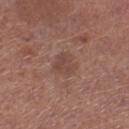This lesion was catalogued during total-body skin photography and was not selected for biopsy.
The lesion is on the right lower leg.
Imaged with white-light lighting.
A female subject about 50 years old.
About 2.5 mm across.
A roughly 15 mm field-of-view crop from a total-body skin photograph.